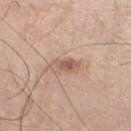Impression: Recorded during total-body skin imaging; not selected for excision or biopsy. Background: The tile uses white-light illumination. Located on the leg. A 15 mm close-up tile from a total-body photography series done for melanoma screening. A male subject, aged 68 to 72.